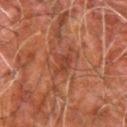| feature | finding |
|---|---|
| workup | imaged on a skin check; not biopsied |
| body site | the left forearm |
| acquisition | total-body-photography crop, ~15 mm field of view |
| tile lighting | cross-polarized |
| patient | male, aged 78 to 82 |